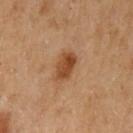The lesion was tiled from a total-body skin photograph and was not biopsied. A female patient, in their 60s. Approximately 3.5 mm at its widest. An algorithmic analysis of the crop reported a lesion area of about 6 mm² and a shape-asymmetry score of about 0.25 (0 = symmetric). It also reported a lesion–skin lightness drop of about 12. The analysis additionally found border irregularity of about 2.5 on a 0–10 scale, a within-lesion color-variation index near 4/10, and radial color variation of about 1.5. A 15 mm close-up tile from a total-body photography series done for melanoma screening. Located on the left upper arm. The tile uses cross-polarized illumination.